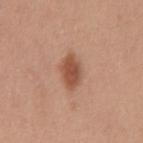notes — no biopsy performed (imaged during a skin exam)
lesion size — ≈4 mm
subject — male, about 50 years old
site — the mid back
imaging modality — ~15 mm tile from a whole-body skin photo
tile lighting — white-light